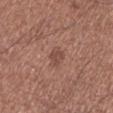Imaged during a routine full-body skin examination; the lesion was not biopsied and no histopathology is available. The lesion is located on the left lower leg. Cropped from a whole-body photographic skin survey; the tile spans about 15 mm. The subject is a male in their 70s.